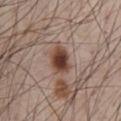The lesion was photographed on a routine skin check and not biopsied; there is no pathology result.
The lesion's longest dimension is about 4 mm.
From the chest.
Imaged with white-light lighting.
A 15 mm close-up tile from a total-body photography series done for melanoma screening.
The total-body-photography lesion software estimated a lesion area of about 8 mm², an outline eccentricity of about 0.7 (0 = round, 1 = elongated), and a symmetry-axis asymmetry near 0.2. The analysis additionally found a lesion–skin lightness drop of about 15 and a normalized border contrast of about 11.5.
A male patient aged 63 to 67.